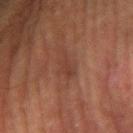Longest diameter approximately 4 mm.
A lesion tile, about 15 mm wide, cut from a 3D total-body photograph.
A male patient, aged around 70.
Automated tile analysis of the lesion measured a footprint of about 4.5 mm².
The lesion is located on the left upper arm.